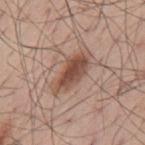{"biopsy_status": "not biopsied; imaged during a skin examination", "site": "mid back", "patient": {"sex": "male", "age_approx": 55}, "image": {"source": "total-body photography crop", "field_of_view_mm": 15}}